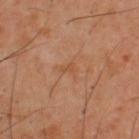Acquisition and patient details: Automated image analysis of the tile measured a lesion color around L≈50 a*≈22 b*≈35 in CIELAB, roughly 5 lightness units darker than nearby skin, and a lesion-to-skin contrast of about 4.5 (normalized; higher = more distinct). The analysis additionally found a color-variation rating of about 0/10. It also reported a classifier nevus-likeness of about 0/100 and lesion-presence confidence of about 100/100. The lesion is on the upper back. A 15 mm close-up tile from a total-body photography series done for melanoma screening. The lesion's longest dimension is about 2.5 mm. A male subject aged approximately 40. The tile uses cross-polarized illumination.